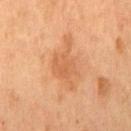Assessment: The lesion was photographed on a routine skin check and not biopsied; there is no pathology result. Image and clinical context: This image is a 15 mm lesion crop taken from a total-body photograph. The lesion is located on the chest. A male subject, aged around 55. An algorithmic analysis of the crop reported a shape eccentricity near 0.85 and a shape-asymmetry score of about 0.45 (0 = symmetric). The software also gave an average lesion color of about L≈56 a*≈24 b*≈38 (CIELAB), a lesion–skin lightness drop of about 8, and a lesion-to-skin contrast of about 6 (normalized; higher = more distinct). It also reported a nevus-likeness score of about 0/100 and a lesion-detection confidence of about 100/100. This is a cross-polarized tile.